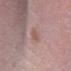A male subject, in their 50s.
A lesion tile, about 15 mm wide, cut from a 3D total-body photograph.
Located on the right lower leg.
The tile uses white-light illumination.
Longest diameter approximately 4 mm.
The total-body-photography lesion software estimated an automated nevus-likeness rating near 5 out of 100 and lesion-presence confidence of about 100/100.